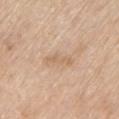Impression: Imaged during a routine full-body skin examination; the lesion was not biopsied and no histopathology is available. Acquisition and patient details: About 3.5 mm across. From the left upper arm. A female patient aged 63 to 67. A roughly 15 mm field-of-view crop from a total-body skin photograph. Captured under white-light illumination.